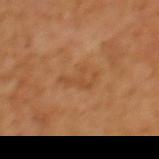Q: Was a biopsy performed?
A: catalogued during a skin exam; not biopsied
Q: What is the lesion's diameter?
A: about 4 mm
Q: What kind of image is this?
A: ~15 mm tile from a whole-body skin photo
Q: What is the anatomic site?
A: the upper back
Q: What are the patient's age and sex?
A: female, aged approximately 40